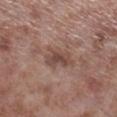The lesion was tiled from a total-body skin photograph and was not biopsied.
Cropped from a whole-body photographic skin survey; the tile spans about 15 mm.
Located on the right lower leg.
The recorded lesion diameter is about 4 mm.
The patient is a male aged 53–57.
The total-body-photography lesion software estimated an outline eccentricity of about 0.8 (0 = round, 1 = elongated). It also reported border irregularity of about 3.5 on a 0–10 scale and a color-variation rating of about 3/10. The analysis additionally found a nevus-likeness score of about 0/100 and a detector confidence of about 100 out of 100 that the crop contains a lesion.
Imaged with white-light lighting.